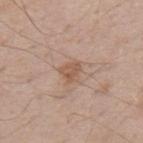<case>
<biopsy_status>not biopsied; imaged during a skin examination</biopsy_status>
<site>mid back</site>
<lesion_size>
  <long_diameter_mm_approx>2.5</long_diameter_mm_approx>
</lesion_size>
<automated_metrics>
  <area_mm2_approx>4.0</area_mm2_approx>
  <eccentricity>0.6</eccentricity>
  <shape_asymmetry>0.4</shape_asymmetry>
  <cielab_L>55</cielab_L>
  <cielab_a>18</cielab_a>
  <cielab_b>29</cielab_b>
  <vs_skin_darker_L>8.0</vs_skin_darker_L>
  <vs_skin_contrast_norm>6.5</vs_skin_contrast_norm>
  <border_irregularity_0_10>3.5</border_irregularity_0_10>
  <color_variation_0_10>2.0</color_variation_0_10>
  <peripheral_color_asymmetry>0.5</peripheral_color_asymmetry>
</automated_metrics>
<patient>
  <sex>male</sex>
  <age_approx>70</age_approx>
</patient>
<image>
  <source>total-body photography crop</source>
  <field_of_view_mm>15</field_of_view_mm>
</image>
<lighting>white-light</lighting>
</case>